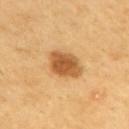notes: no biopsy performed (imaged during a skin exam).